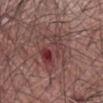<record>
<biopsy_status>not biopsied; imaged during a skin examination</biopsy_status>
<site>left forearm</site>
<lighting>white-light</lighting>
<automated_metrics>
  <cielab_L>38</cielab_L>
  <cielab_a>24</cielab_a>
  <cielab_b>19</cielab_b>
  <vs_skin_darker_L>8.0</vs_skin_darker_L>
  <vs_skin_contrast_norm>7.0</vs_skin_contrast_norm>
</automated_metrics>
<lesion_size>
  <long_diameter_mm_approx>5.0</long_diameter_mm_approx>
</lesion_size>
<image>
  <source>total-body photography crop</source>
  <field_of_view_mm>15</field_of_view_mm>
</image>
<patient>
  <sex>male</sex>
  <age_approx>60</age_approx>
</patient>
</record>